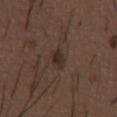• biopsy status: imaged on a skin check; not biopsied
• size: ~2.5 mm (longest diameter)
• anatomic site: the abdomen
• subject: male, about 50 years old
• imaging modality: ~15 mm tile from a whole-body skin photo
• tile lighting: white-light illumination